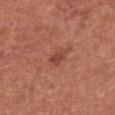Q: Was this lesion biopsied?
A: total-body-photography surveillance lesion; no biopsy
Q: Lesion location?
A: the chest
Q: What lighting was used for the tile?
A: white-light illumination
Q: What are the patient's age and sex?
A: female, in their 50s
Q: What is the imaging modality?
A: ~15 mm tile from a whole-body skin photo
Q: How large is the lesion?
A: ~3 mm (longest diameter)